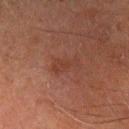Case summary:
* tile lighting · cross-polarized illumination
* image-analysis metrics · a shape eccentricity near 0.85; a border-irregularity index near 5/10, internal color variation of about 1 on a 0–10 scale, and a peripheral color-asymmetry measure near 0.5; a nevus-likeness score of about 5/100 and a lesion-detection confidence of about 100/100
* diameter · about 3 mm
* image · total-body-photography crop, ~15 mm field of view
* patient · male, about 60 years old
* site · the left arm